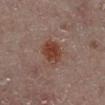Impression: Imaged during a routine full-body skin examination; the lesion was not biopsied and no histopathology is available. Image and clinical context: A male patient about 60 years old. A region of skin cropped from a whole-body photographic capture, roughly 15 mm wide. The lesion is on the right lower leg. The lesion's longest dimension is about 3 mm.